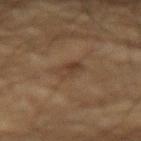– biopsy status · catalogued during a skin exam; not biopsied
– site · the right upper arm
– image · 15 mm crop, total-body photography
– subject · male, aged approximately 85 or older
– diameter · ~3 mm (longest diameter)
– automated lesion analysis · a lesion-detection confidence of about 100/100
– illumination · cross-polarized